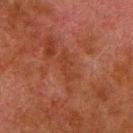  biopsy_status: not biopsied; imaged during a skin examination
  image:
    source: total-body photography crop
    field_of_view_mm: 15
  site: right lower leg
  patient:
    sex: male
    age_approx: 80
  lighting: cross-polarized
  lesion_size:
    long_diameter_mm_approx: 3.5